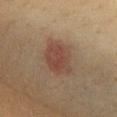Q: Was a biopsy performed?
A: total-body-photography surveillance lesion; no biopsy
Q: Patient demographics?
A: female, aged 38–42
Q: Where on the body is the lesion?
A: the chest
Q: How was this image acquired?
A: ~15 mm crop, total-body skin-cancer survey
Q: How was the tile lit?
A: cross-polarized
Q: Lesion size?
A: about 5 mm